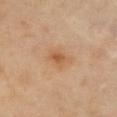workup: catalogued during a skin exam; not biopsied
acquisition: ~15 mm crop, total-body skin-cancer survey
illumination: cross-polarized
patient: female, aged approximately 40
image-analysis metrics: an area of roughly 4.5 mm², a shape eccentricity near 0.7, and a symmetry-axis asymmetry near 0.3; an average lesion color of about L≈56 a*≈20 b*≈37 (CIELAB) and a lesion–skin lightness drop of about 8
site: the chest